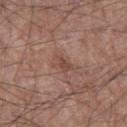- patient · male, about 60 years old
- image source · ~15 mm crop, total-body skin-cancer survey
- size · about 2.5 mm
- lighting · white-light illumination
- anatomic site · the left thigh